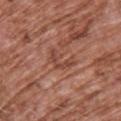notes=imaged on a skin check; not biopsied | patient=male, aged 73–77 | lighting=white-light | lesion size=~3.5 mm (longest diameter) | image source=15 mm crop, total-body photography | body site=the chest | TBP lesion metrics=a lesion color around L≈45 a*≈24 b*≈29 in CIELAB, a lesion–skin lightness drop of about 8, and a normalized lesion–skin contrast near 6; a border-irregularity index near 9/10, internal color variation of about 0 on a 0–10 scale, and radial color variation of about 0.